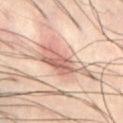Imaged during a routine full-body skin examination; the lesion was not biopsied and no histopathology is available. On the abdomen. A 15 mm close-up tile from a total-body photography series done for melanoma screening. The patient is a male aged approximately 50. Measured at roughly 8 mm in maximum diameter. This is a cross-polarized tile.